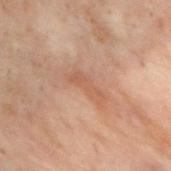Findings:
* biopsy status · imaged on a skin check; not biopsied
* imaging modality · total-body-photography crop, ~15 mm field of view
* automated lesion analysis · a border-irregularity rating of about 5/10, internal color variation of about 0.5 on a 0–10 scale, and a peripheral color-asymmetry measure near 0; an automated nevus-likeness rating near 0 out of 100
* body site · the upper back
* patient · male, roughly 30 years of age
* illumination · cross-polarized